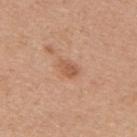biopsy_status: not biopsied; imaged during a skin examination
lesion_size:
  long_diameter_mm_approx: 2.5
lighting: white-light
automated_metrics:
  area_mm2_approx: 3.5
  eccentricity: 0.75
  shape_asymmetry: 0.3
  border_irregularity_0_10: 3.0
  color_variation_0_10: 2.0
  peripheral_color_asymmetry: 0.5
  nevus_likeness_0_100: 30
  lesion_detection_confidence_0_100: 100
site: upper back
patient:
  sex: female
  age_approx: 40
image:
  source: total-body photography crop
  field_of_view_mm: 15